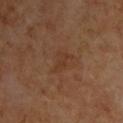The lesion was tiled from a total-body skin photograph and was not biopsied. The subject is a male aged around 65. On the chest. The recorded lesion diameter is about 2.5 mm. A roughly 15 mm field-of-view crop from a total-body skin photograph. Automated tile analysis of the lesion measured a lesion area of about 3.5 mm² and two-axis asymmetry of about 0.4. And it measured a lesion-detection confidence of about 100/100. Captured under cross-polarized illumination.